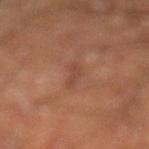– biopsy status · no biopsy performed (imaged during a skin exam)
– image source · 15 mm crop, total-body photography
– lighting · cross-polarized illumination
– subject · male, aged 58–62
– lesion diameter · about 3.5 mm
– image-analysis metrics · border irregularity of about 6 on a 0–10 scale and radial color variation of about 0
– site · the left lower leg